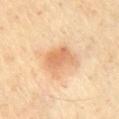<lesion>
<biopsy_status>not biopsied; imaged during a skin examination</biopsy_status>
<image>
  <source>total-body photography crop</source>
  <field_of_view_mm>15</field_of_view_mm>
</image>
<lesion_size>
  <long_diameter_mm_approx>4.0</long_diameter_mm_approx>
</lesion_size>
<site>chest</site>
<automated_metrics>
  <nevus_likeness_0_100>90</nevus_likeness_0_100>
  <lesion_detection_confidence_0_100>100</lesion_detection_confidence_0_100>
</automated_metrics>
<patient>
  <sex>male</sex>
  <age_approx>70</age_approx>
</patient>
<lighting>cross-polarized</lighting>
</lesion>